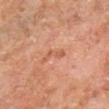* illumination: cross-polarized
* body site: the right lower leg
* patient: male, aged around 65
* size: about 3 mm
* acquisition: ~15 mm crop, total-body skin-cancer survey